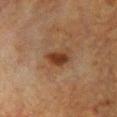Context: This is a cross-polarized tile. A female subject, aged around 55. About 3.5 mm across. A region of skin cropped from a whole-body photographic capture, roughly 15 mm wide. The lesion-visualizer software estimated an area of roughly 5.5 mm², a shape eccentricity near 0.75, and a shape-asymmetry score of about 0.25 (0 = symmetric). Located on the front of the torso.